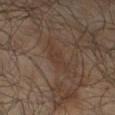No biopsy was performed on this lesion — it was imaged during a full skin examination and was not determined to be concerning. From the leg. A male patient roughly 65 years of age. A close-up tile cropped from a whole-body skin photograph, about 15 mm across. Longest diameter approximately 4.5 mm.